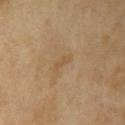| key | value |
|---|---|
| workup | imaged on a skin check; not biopsied |
| acquisition | ~15 mm tile from a whole-body skin photo |
| patient | female, about 60 years old |
| size | about 2.5 mm |
| TBP lesion metrics | a lesion color around L≈48 a*≈14 b*≈33 in CIELAB and a normalized border contrast of about 4.5; internal color variation of about 0 on a 0–10 scale and peripheral color asymmetry of about 0 |
| location | the right upper arm |
| tile lighting | cross-polarized illumination |